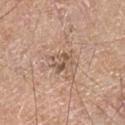The lesion was photographed on a routine skin check and not biopsied; there is no pathology result.